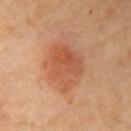Impression: No biopsy was performed on this lesion — it was imaged during a full skin examination and was not determined to be concerning. Background: A lesion tile, about 15 mm wide, cut from a 3D total-body photograph. Measured at roughly 6 mm in maximum diameter. The subject is a female in their 60s. From the left arm.